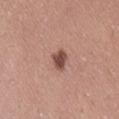Assessment:
This lesion was catalogued during total-body skin photography and was not selected for biopsy.
Background:
A female subject, roughly 40 years of age. On the lower back. This image is a 15 mm lesion crop taken from a total-body photograph.